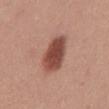Impression:
Imaged during a routine full-body skin examination; the lesion was not biopsied and no histopathology is available.
Context:
Longest diameter approximately 5 mm. Imaged with white-light lighting. The lesion is on the mid back. Cropped from a whole-body photographic skin survey; the tile spans about 15 mm. The patient is a female aged 23 to 27.